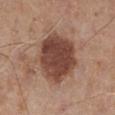Part of a total-body skin-imaging series; this lesion was reviewed on a skin check and was not flagged for biopsy. Automated tile analysis of the lesion measured an average lesion color of about L≈44 a*≈21 b*≈26 (CIELAB) and roughly 15 lightness units darker than nearby skin. It also reported a border-irregularity rating of about 1.5/10 and internal color variation of about 4 on a 0–10 scale. The patient is a male roughly 55 years of age. From the left lower leg. This image is a 15 mm lesion crop taken from a total-body photograph. This is a white-light tile.